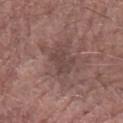Q: What is the anatomic site?
A: the arm
Q: Lesion size?
A: ~3 mm (longest diameter)
Q: What is the imaging modality?
A: ~15 mm tile from a whole-body skin photo
Q: What are the patient's age and sex?
A: male, approximately 70 years of age
Q: Automated lesion metrics?
A: border irregularity of about 4 on a 0–10 scale, a color-variation rating of about 1/10, and a peripheral color-asymmetry measure near 0